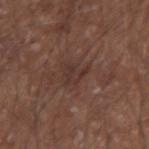notes — total-body-photography surveillance lesion; no biopsy
tile lighting — white-light illumination
automated metrics — a mean CIELAB color near L≈33 a*≈18 b*≈21, about 6 CIELAB-L* units darker than the surrounding skin, and a normalized border contrast of about 6; a border-irregularity index near 6.5/10 and a within-lesion color-variation index near 1.5/10
patient — male, in their mid- to late 60s
imaging modality — ~15 mm tile from a whole-body skin photo
size — about 3 mm
site — the arm